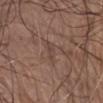biopsy_status: not biopsied; imaged during a skin examination
lesion_size:
  long_diameter_mm_approx: 2.5
site: chest
image:
  source: total-body photography crop
  field_of_view_mm: 15
patient:
  sex: male
  age_approx: 75